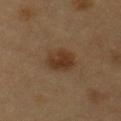body site: the chest | imaging modality: ~15 mm crop, total-body skin-cancer survey | lesion diameter: ≈3.5 mm | TBP lesion metrics: an area of roughly 8.5 mm², an outline eccentricity of about 0.6 (0 = round, 1 = elongated), and two-axis asymmetry of about 0.2; an average lesion color of about L≈34 a*≈16 b*≈29 (CIELAB), roughly 8 lightness units darker than nearby skin, and a normalized border contrast of about 8.5 | subject: male, aged 53–57 | lighting: cross-polarized.